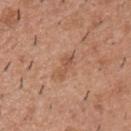Recorded during total-body skin imaging; not selected for excision or biopsy.
A male patient, aged around 40.
Imaged with white-light lighting.
The lesion is on the upper back.
A region of skin cropped from a whole-body photographic capture, roughly 15 mm wide.
Measured at roughly 3 mm in maximum diameter.
The lesion-visualizer software estimated about 8 CIELAB-L* units darker than the surrounding skin. The analysis additionally found internal color variation of about 0 on a 0–10 scale and radial color variation of about 0.